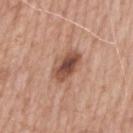{"biopsy_status": "not biopsied; imaged during a skin examination", "automated_metrics": {"area_mm2_approx": 9.5, "shape_asymmetry": 0.2, "nevus_likeness_0_100": 80, "lesion_detection_confidence_0_100": 100}, "site": "arm", "lighting": "white-light", "image": {"source": "total-body photography crop", "field_of_view_mm": 15}, "patient": {"sex": "male", "age_approx": 60}, "lesion_size": {"long_diameter_mm_approx": 4.0}}